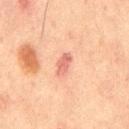| feature | finding |
|---|---|
| site | the mid back |
| automated metrics | a mean CIELAB color near L≈53 a*≈24 b*≈26, roughly 9 lightness units darker than nearby skin, and a normalized lesion–skin contrast near 6.5; an automated nevus-likeness rating near 15 out of 100 and lesion-presence confidence of about 100/100 |
| subject | male, aged 63–67 |
| image source | ~15 mm tile from a whole-body skin photo |
| tile lighting | cross-polarized |